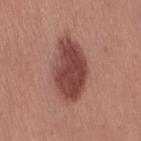A female subject, roughly 35 years of age.
An algorithmic analysis of the crop reported two-axis asymmetry of about 0.2. The software also gave a normalized border contrast of about 10.5. The software also gave border irregularity of about 2 on a 0–10 scale and radial color variation of about 1. The analysis additionally found a classifier nevus-likeness of about 85/100.
The lesion is on the left thigh.
The recorded lesion diameter is about 7 mm.
A region of skin cropped from a whole-body photographic capture, roughly 15 mm wide.